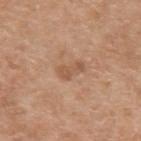Clinical summary: The total-body-photography lesion software estimated a mean CIELAB color near L≈55 a*≈20 b*≈33, a lesion–skin lightness drop of about 8, and a normalized lesion–skin contrast near 5.5. A 15 mm close-up extracted from a 3D total-body photography capture. From the left upper arm. The tile uses white-light illumination. The lesion's longest dimension is about 3 mm. The patient is a male aged approximately 60.